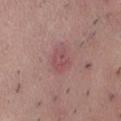Q: Was this lesion biopsied?
A: total-body-photography surveillance lesion; no biopsy
Q: Lesion location?
A: the abdomen
Q: What are the patient's age and sex?
A: female, in their mid-30s
Q: What is the imaging modality?
A: ~15 mm tile from a whole-body skin photo